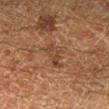Acquisition and patient details:
A close-up tile cropped from a whole-body skin photograph, about 15 mm across. The subject is a male aged around 60. From the right lower leg. Automated tile analysis of the lesion measured an area of roughly 4.5 mm² and a symmetry-axis asymmetry near 0.45. And it measured a mean CIELAB color near L≈32 a*≈16 b*≈25 and a normalized lesion–skin contrast near 6. The software also gave a nevus-likeness score of about 0/100 and lesion-presence confidence of about 100/100.